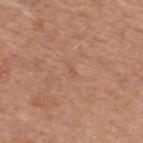The lesion was tiled from a total-body skin photograph and was not biopsied. The patient is a male in their 50s. This image is a 15 mm lesion crop taken from a total-body photograph. Located on the upper back.